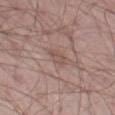{"biopsy_status": "not biopsied; imaged during a skin examination", "image": {"source": "total-body photography crop", "field_of_view_mm": 15}, "site": "left thigh", "patient": {"sex": "male", "age_approx": 65}}